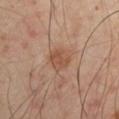{
  "lighting": "cross-polarized",
  "image": {
    "source": "total-body photography crop",
    "field_of_view_mm": 15
  },
  "patient": {
    "sex": "male",
    "age_approx": 50
  },
  "site": "left arm"
}